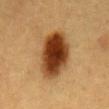notes = imaged on a skin check; not biopsied
lesion size = ≈7 mm
automated metrics = an average lesion color of about L≈34 a*≈19 b*≈32 (CIELAB), a lesion–skin lightness drop of about 16, and a normalized lesion–skin contrast near 14
location = the mid back
tile lighting = cross-polarized illumination
subject = female, aged around 55
imaging modality = ~15 mm crop, total-body skin-cancer survey